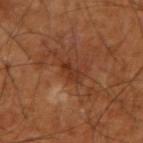| field | value |
|---|---|
| biopsy status | total-body-photography surveillance lesion; no biopsy |
| subject | male, aged around 60 |
| site | the right upper arm |
| lesion diameter | ~2.5 mm (longest diameter) |
| image source | 15 mm crop, total-body photography |
| automated metrics | an area of roughly 2.5 mm², an outline eccentricity of about 0.85 (0 = round, 1 = elongated), and a shape-asymmetry score of about 0.35 (0 = symmetric); an average lesion color of about L≈32 a*≈23 b*≈31 (CIELAB), about 8 CIELAB-L* units darker than the surrounding skin, and a normalized lesion–skin contrast near 7 |
| illumination | cross-polarized illumination |